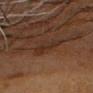- biopsy status · total-body-photography surveillance lesion; no biopsy
- subject · male, about 60 years old
- site · the head or neck
- image source · ~15 mm tile from a whole-body skin photo
- automated lesion analysis · a mean CIELAB color near L≈23 a*≈15 b*≈22, a lesion–skin lightness drop of about 5, and a lesion-to-skin contrast of about 6 (normalized; higher = more distinct); border irregularity of about 3.5 on a 0–10 scale and peripheral color asymmetry of about 1; a detector confidence of about 75 out of 100 that the crop contains a lesion
- lesion diameter · ~3.5 mm (longest diameter)